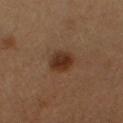Recorded during total-body skin imaging; not selected for excision or biopsy.
Measured at roughly 3 mm in maximum diameter.
A lesion tile, about 15 mm wide, cut from a 3D total-body photograph.
The lesion is located on the right upper arm.
Automated tile analysis of the lesion measured an automated nevus-likeness rating near 100 out of 100 and a lesion-detection confidence of about 100/100.
A male patient, aged approximately 50.